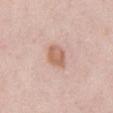Findings:
• illumination: white-light illumination
• automated metrics: a lesion area of about 5.5 mm², a shape eccentricity near 0.65, and a shape-asymmetry score of about 0.25 (0 = symmetric); an automated nevus-likeness rating near 40 out of 100 and a lesion-detection confidence of about 100/100
• imaging modality: ~15 mm tile from a whole-body skin photo
• patient: female, approximately 60 years of age
• diameter: about 3 mm
• body site: the abdomen